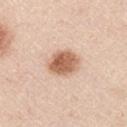Context:
The patient is a male about 45 years old. A roughly 15 mm field-of-view crop from a total-body skin photograph. The lesion is on the left upper arm.